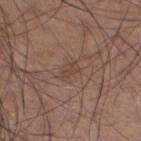Impression:
This lesion was catalogued during total-body skin photography and was not selected for biopsy.
Context:
Approximately 2.5 mm at its widest. A lesion tile, about 15 mm wide, cut from a 3D total-body photograph. An algorithmic analysis of the crop reported an area of roughly 2.5 mm² and two-axis asymmetry of about 0.4. It also reported a border-irregularity rating of about 4.5/10, a color-variation rating of about 0/10, and radial color variation of about 0. The lesion is on the left lower leg. Imaged with white-light lighting. A male subject approximately 55 years of age.